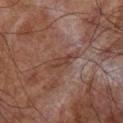biopsy status: catalogued during a skin exam; not biopsied | size: ~3 mm (longest diameter) | body site: the right lower leg | image source: ~15 mm crop, total-body skin-cancer survey | subject: male, aged around 70.